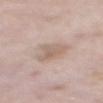Part of a total-body skin-imaging series; this lesion was reviewed on a skin check and was not flagged for biopsy.
From the mid back.
Imaged with white-light lighting.
A male patient aged 58–62.
Automated tile analysis of the lesion measured border irregularity of about 3.5 on a 0–10 scale and a color-variation rating of about 2.5/10. The analysis additionally found a nevus-likeness score of about 0/100.
This image is a 15 mm lesion crop taken from a total-body photograph.
Approximately 4 mm at its widest.